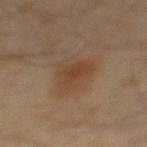Clinical impression:
The lesion was tiled from a total-body skin photograph and was not biopsied.
Clinical summary:
The lesion is on the mid back. The tile uses cross-polarized illumination. Cropped from a total-body skin-imaging series; the visible field is about 15 mm. A male subject, aged around 40.